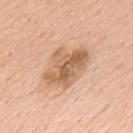notes = total-body-photography surveillance lesion; no biopsy | subject = male, roughly 60 years of age | tile lighting = white-light | imaging modality = ~15 mm crop, total-body skin-cancer survey | location = the upper back.